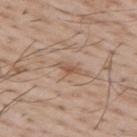Automated image analysis of the tile measured a footprint of about 2.5 mm², an eccentricity of roughly 0.9, and a shape-asymmetry score of about 0.35 (0 = symmetric). A male subject, aged approximately 65. About 2.5 mm across. Imaged with white-light lighting. A region of skin cropped from a whole-body photographic capture, roughly 15 mm wide. On the upper back.